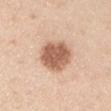Recorded during total-body skin imaging; not selected for excision or biopsy. The lesion is located on the right upper arm. Automated image analysis of the tile measured a lesion area of about 14 mm² and a shape eccentricity near 0.4. A lesion tile, about 15 mm wide, cut from a 3D total-body photograph. A male patient, approximately 35 years of age. Approximately 4.5 mm at its widest.